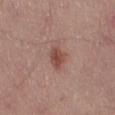The lesion was photographed on a routine skin check and not biopsied; there is no pathology result. A 15 mm crop from a total-body photograph taken for skin-cancer surveillance. About 3 mm across. Automated tile analysis of the lesion measured a lesion color around L≈48 a*≈22 b*≈25 in CIELAB, about 10 CIELAB-L* units darker than the surrounding skin, and a lesion-to-skin contrast of about 7.5 (normalized; higher = more distinct). The analysis additionally found an automated nevus-likeness rating near 85 out of 100 and a lesion-detection confidence of about 100/100. Imaged with white-light lighting. On the left thigh. A male patient, aged approximately 55.